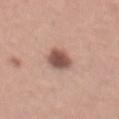Impression: The lesion was tiled from a total-body skin photograph and was not biopsied. Clinical summary: The subject is a male in their mid- to late 40s. A close-up tile cropped from a whole-body skin photograph, about 15 mm across. Located on the mid back. The lesion's longest dimension is about 3.5 mm.